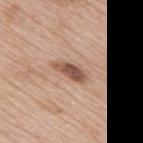<case>
<biopsy_status>not biopsied; imaged during a skin examination</biopsy_status>
<automated_metrics>
  <area_mm2_approx>6.0</area_mm2_approx>
  <border_irregularity_0_10>3.0</border_irregularity_0_10>
  <color_variation_0_10>4.5</color_variation_0_10>
  <peripheral_color_asymmetry>1.5</peripheral_color_asymmetry>
</automated_metrics>
<site>upper back</site>
<image>
  <source>total-body photography crop</source>
  <field_of_view_mm>15</field_of_view_mm>
</image>
<patient>
  <sex>male</sex>
  <age_approx>80</age_approx>
</patient>
<lesion_size>
  <long_diameter_mm_approx>4.0</long_diameter_mm_approx>
</lesion_size>
<lighting>white-light</lighting>
</case>